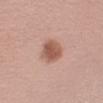Image and clinical context: Located on the left upper arm. A 15 mm close-up extracted from a 3D total-body photography capture. Captured under white-light illumination. A female patient, approximately 45 years of age. Automated tile analysis of the lesion measured an area of roughly 8 mm², a shape eccentricity near 0.55, and a shape-asymmetry score of about 0.15 (0 = symmetric). It also reported a classifier nevus-likeness of about 100/100 and lesion-presence confidence of about 100/100.